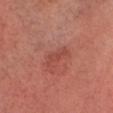This lesion was catalogued during total-body skin photography and was not selected for biopsy. Imaged with cross-polarized lighting. From the head or neck. Cropped from a total-body skin-imaging series; the visible field is about 15 mm. A male patient, aged 48 to 52. The lesion-visualizer software estimated a footprint of about 3.5 mm², an outline eccentricity of about 0.95 (0 = round, 1 = elongated), and a shape-asymmetry score of about 0.45 (0 = symmetric). The software also gave a mean CIELAB color near L≈46 a*≈29 b*≈28 and a lesion–skin lightness drop of about 7.